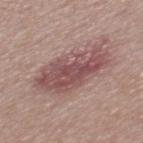Q: Was this lesion biopsied?
A: no biopsy performed (imaged during a skin exam)
Q: Automated lesion metrics?
A: a border-irregularity rating of about 2.5/10, a color-variation rating of about 5/10, and radial color variation of about 2; a classifier nevus-likeness of about 20/100 and a detector confidence of about 100 out of 100 that the crop contains a lesion
Q: How was this image acquired?
A: ~15 mm tile from a whole-body skin photo
Q: What are the patient's age and sex?
A: male, aged around 55
Q: What lighting was used for the tile?
A: white-light illumination
Q: What is the anatomic site?
A: the mid back